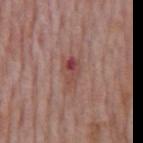Longest diameter approximately 4 mm. The lesion is on the mid back. The lesion-visualizer software estimated a footprint of about 5.5 mm², an outline eccentricity of about 0.85 (0 = round, 1 = elongated), and a shape-asymmetry score of about 0.3 (0 = symmetric). It also reported a border-irregularity rating of about 3/10 and peripheral color asymmetry of about 4. The software also gave an automated nevus-likeness rating near 0 out of 100 and a lesion-detection confidence of about 100/100. The tile uses white-light illumination. A 15 mm close-up extracted from a 3D total-body photography capture. The subject is a male approximately 75 years of age.